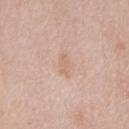patient: male, approximately 60 years of age; tile lighting: white-light illumination; anatomic site: the chest; image source: total-body-photography crop, ~15 mm field of view.